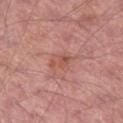The lesion was tiled from a total-body skin photograph and was not biopsied. This is a white-light tile. The lesion is on the left thigh. The patient is a male aged around 65. A 15 mm close-up extracted from a 3D total-body photography capture.